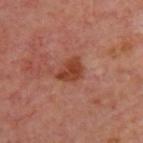The lesion was tiled from a total-body skin photograph and was not biopsied.
The patient is aged approximately 55.
The lesion is on the upper back.
Imaged with cross-polarized lighting.
Cropped from a total-body skin-imaging series; the visible field is about 15 mm.
The recorded lesion diameter is about 3 mm.